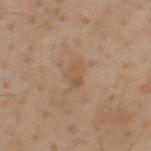Case summary:
- follow-up — no biopsy performed (imaged during a skin exam)
- subject — male, aged approximately 55
- location — the upper back
- lesion diameter — about 2.5 mm
- automated metrics — a mean CIELAB color near L≈53 a*≈16 b*≈33 and a normalized border contrast of about 5.5; a border-irregularity index near 3.5/10, a color-variation rating of about 2/10, and peripheral color asymmetry of about 0.5
- imaging modality — ~15 mm crop, total-body skin-cancer survey